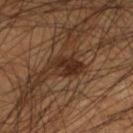Recorded during total-body skin imaging; not selected for excision or biopsy.
A male patient aged around 45.
The lesion is on the left lower leg.
A region of skin cropped from a whole-body photographic capture, roughly 15 mm wide.
Imaged with cross-polarized lighting.